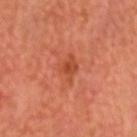biopsy status: no biopsy performed (imaged during a skin exam)
site: the head or neck
subject: male, approximately 65 years of age
tile lighting: cross-polarized
imaging modality: 15 mm crop, total-body photography
TBP lesion metrics: an outline eccentricity of about 0.5 (0 = round, 1 = elongated) and a symmetry-axis asymmetry near 0.3; a lesion color around L≈47 a*≈32 b*≈37 in CIELAB, roughly 8 lightness units darker than nearby skin, and a normalized lesion–skin contrast near 6; an automated nevus-likeness rating near 0 out of 100